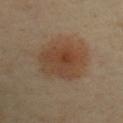{"biopsy_status": "not biopsied; imaged during a skin examination", "image": {"source": "total-body photography crop", "field_of_view_mm": 15}, "patient": {"sex": "male", "age_approx": 40}, "site": "front of the torso", "lesion_size": {"long_diameter_mm_approx": 6.0}}